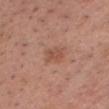biopsy_status: not biopsied; imaged during a skin examination
lesion_size:
  long_diameter_mm_approx: 2.5
image:
  source: total-body photography crop
  field_of_view_mm: 15
site: chest
lighting: white-light
patient:
  sex: male
  age_approx: 55